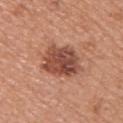Captured during whole-body skin photography for melanoma surveillance; the lesion was not biopsied. The subject is a female roughly 35 years of age. Cropped from a total-body skin-imaging series; the visible field is about 15 mm. The lesion is located on the upper back.